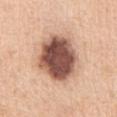Q: Was this lesion biopsied?
A: total-body-photography surveillance lesion; no biopsy
Q: How large is the lesion?
A: ~6.5 mm (longest diameter)
Q: What lighting was used for the tile?
A: white-light
Q: What is the anatomic site?
A: the abdomen
Q: What is the imaging modality?
A: ~15 mm crop, total-body skin-cancer survey
Q: Patient demographics?
A: female, about 55 years old